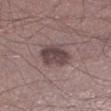| feature | finding |
|---|---|
| biopsy status | total-body-photography surveillance lesion; no biopsy |
| location | the left lower leg |
| TBP lesion metrics | an average lesion color of about L≈43 a*≈16 b*≈15 (CIELAB) and a normalized border contrast of about 9 |
| subject | male, approximately 35 years of age |
| imaging modality | 15 mm crop, total-body photography |
| size | ~4 mm (longest diameter) |
| tile lighting | white-light illumination |